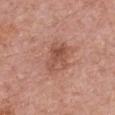Impression:
The lesion was tiled from a total-body skin photograph and was not biopsied.
Context:
A female subject aged approximately 60. The lesion's longest dimension is about 4 mm. On the chest. Imaged with white-light lighting. A roughly 15 mm field-of-view crop from a total-body skin photograph.